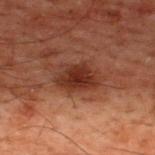{
  "biopsy_status": "not biopsied; imaged during a skin examination",
  "image": {
    "source": "total-body photography crop",
    "field_of_view_mm": 15
  },
  "patient": {
    "sex": "male",
    "age_approx": 60
  },
  "site": "upper back"
}